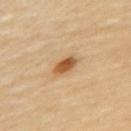Assessment:
Part of a total-body skin-imaging series; this lesion was reviewed on a skin check and was not flagged for biopsy.
Image and clinical context:
This image is a 15 mm lesion crop taken from a total-body photograph. The lesion is located on the upper back. The patient is a male roughly 70 years of age.